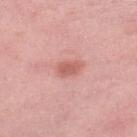Case summary:
* image · 15 mm crop, total-body photography
* site · the left lower leg
* patient · female, aged around 40